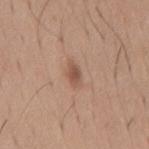Imaged during a routine full-body skin examination; the lesion was not biopsied and no histopathology is available. A region of skin cropped from a whole-body photographic capture, roughly 15 mm wide. On the mid back. A male subject aged 63 to 67.